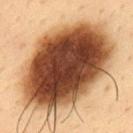A male subject roughly 35 years of age. A region of skin cropped from a whole-body photographic capture, roughly 15 mm wide. Located on the upper back. The total-body-photography lesion software estimated a classifier nevus-likeness of about 100/100. Captured under cross-polarized illumination. Approximately 13.5 mm at its widest.